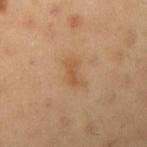The lesion was photographed on a routine skin check and not biopsied; there is no pathology result.
A 15 mm crop from a total-body photograph taken for skin-cancer surveillance.
Automated tile analysis of the lesion measured a lesion color around L≈52 a*≈20 b*≈35 in CIELAB, roughly 7 lightness units darker than nearby skin, and a normalized border contrast of about 6. It also reported border irregularity of about 4 on a 0–10 scale, a within-lesion color-variation index near 1/10, and radial color variation of about 0.5.
About 3 mm across.
Located on the arm.
The tile uses cross-polarized illumination.
The patient is a male roughly 55 years of age.